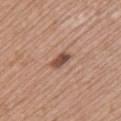Findings:
• follow-up — no biopsy performed (imaged during a skin exam)
• automated lesion analysis — a lesion area of about 3.5 mm², a shape eccentricity near 0.85, and two-axis asymmetry of about 0.2; lesion-presence confidence of about 100/100
• patient — female, roughly 60 years of age
• illumination — white-light illumination
• image source — ~15 mm crop, total-body skin-cancer survey
• size — about 2.5 mm
• anatomic site — the back